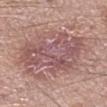The lesion was tiled from a total-body skin photograph and was not biopsied. Cropped from a whole-body photographic skin survey; the tile spans about 15 mm. This is a white-light tile. The lesion is located on the right lower leg. Automated image analysis of the tile measured a mean CIELAB color near L≈53 a*≈22 b*≈19, roughly 9 lightness units darker than nearby skin, and a normalized border contrast of about 7. It also reported a border-irregularity index near 5.5/10 and internal color variation of about 5 on a 0–10 scale. A male patient approximately 60 years of age. Measured at roughly 8 mm in maximum diameter.